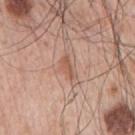Part of a total-body skin-imaging series; this lesion was reviewed on a skin check and was not flagged for biopsy. Approximately 3 mm at its widest. A roughly 15 mm field-of-view crop from a total-body skin photograph. The lesion is located on the arm. Automated tile analysis of the lesion measured an area of roughly 3.5 mm², an eccentricity of roughly 0.9, and two-axis asymmetry of about 0.45. And it measured border irregularity of about 5 on a 0–10 scale, a within-lesion color-variation index near 0.5/10, and peripheral color asymmetry of about 0. A male subject, about 65 years old. Captured under white-light illumination.